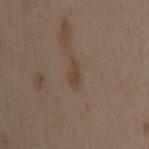Clinical impression:
The lesion was photographed on a routine skin check and not biopsied; there is no pathology result.
Image and clinical context:
On the mid back. The recorded lesion diameter is about 3 mm. A lesion tile, about 15 mm wide, cut from a 3D total-body photograph. The patient is a female approximately 35 years of age. Captured under white-light illumination.